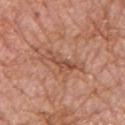Assessment: Captured during whole-body skin photography for melanoma surveillance; the lesion was not biopsied. Image and clinical context: This is a white-light tile. A region of skin cropped from a whole-body photographic capture, roughly 15 mm wide. On the chest. A male patient, aged 53–57. Automated tile analysis of the lesion measured a lesion color around L≈52 a*≈24 b*≈32 in CIELAB, roughly 9 lightness units darker than nearby skin, and a normalized lesion–skin contrast near 6.